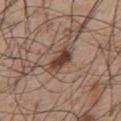| key | value |
|---|---|
| biopsy status | no biopsy performed (imaged during a skin exam) |
| diameter | ~4 mm (longest diameter) |
| site | the chest |
| lighting | white-light illumination |
| subject | male, aged 43–47 |
| image source | 15 mm crop, total-body photography |